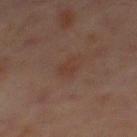Imaged during a routine full-body skin examination; the lesion was not biopsied and no histopathology is available. The lesion is located on the back. Captured under cross-polarized illumination. The subject is a male aged around 60. Automated tile analysis of the lesion measured a border-irregularity index near 4.5/10, internal color variation of about 1 on a 0–10 scale, and radial color variation of about 0.5. The analysis additionally found an automated nevus-likeness rating near 5 out of 100 and a detector confidence of about 100 out of 100 that the crop contains a lesion. The recorded lesion diameter is about 2.5 mm. A region of skin cropped from a whole-body photographic capture, roughly 15 mm wide.